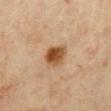notes = no biopsy performed (imaged during a skin exam) | automated metrics = an eccentricity of roughly 0.6 and two-axis asymmetry of about 0.15; an automated nevus-likeness rating near 100 out of 100 and a lesion-detection confidence of about 100/100 | site = the mid back | size = ~3 mm (longest diameter) | image source = ~15 mm tile from a whole-body skin photo | lighting = cross-polarized illumination | patient = male, in their mid- to late 40s.